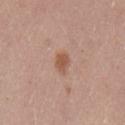Assessment: The lesion was photographed on a routine skin check and not biopsied; there is no pathology result. Clinical summary: Approximately 2.5 mm at its widest. A male subject about 25 years old. A region of skin cropped from a whole-body photographic capture, roughly 15 mm wide. Located on the chest. Imaged with white-light lighting.